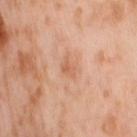This is a cross-polarized tile. Measured at roughly 3 mm in maximum diameter. On the right thigh. A 15 mm close-up tile from a total-body photography series done for melanoma screening. The subject is a female roughly 55 years of age.